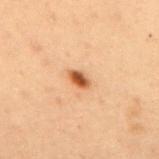| key | value |
|---|---|
| workup | total-body-photography surveillance lesion; no biopsy |
| acquisition | 15 mm crop, total-body photography |
| lesion diameter | about 2.5 mm |
| patient | male, aged around 55 |
| site | the upper back |